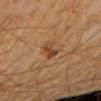biopsy status: total-body-photography surveillance lesion; no biopsy
lighting: cross-polarized illumination
size: about 3.5 mm
image: total-body-photography crop, ~15 mm field of view
automated lesion analysis: a lesion area of about 4 mm², an outline eccentricity of about 0.9 (0 = round, 1 = elongated), and a shape-asymmetry score of about 0.45 (0 = symmetric); an average lesion color of about L≈38 a*≈19 b*≈31 (CIELAB), roughly 9 lightness units darker than nearby skin, and a lesion-to-skin contrast of about 7.5 (normalized; higher = more distinct)
anatomic site: the left arm
subject: male, aged 53 to 57